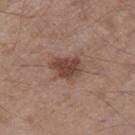{"biopsy_status": "not biopsied; imaged during a skin examination", "image": {"source": "total-body photography crop", "field_of_view_mm": 15}, "site": "leg", "lesion_size": {"long_diameter_mm_approx": 3.5}, "patient": {"sex": "male", "age_approx": 55}, "automated_metrics": {"color_variation_0_10": 3.0, "peripheral_color_asymmetry": 1.0, "nevus_likeness_0_100": 90, "lesion_detection_confidence_0_100": 100}}